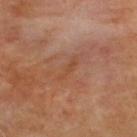Q: Was a biopsy performed?
A: no biopsy performed (imaged during a skin exam)
Q: What is the anatomic site?
A: the upper back
Q: Patient demographics?
A: male, about 70 years old
Q: What lighting was used for the tile?
A: cross-polarized
Q: What is the imaging modality?
A: 15 mm crop, total-body photography